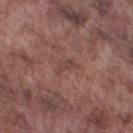No biopsy was performed on this lesion — it was imaged during a full skin examination and was not determined to be concerning.